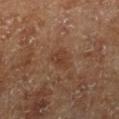Clinical impression: The lesion was photographed on a routine skin check and not biopsied; there is no pathology result. Clinical summary: The lesion is located on the right lower leg. A region of skin cropped from a whole-body photographic capture, roughly 15 mm wide. This is a cross-polarized tile. A male subject, aged around 70. The recorded lesion diameter is about 2.5 mm.